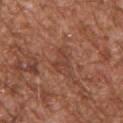Clinical impression: Imaged during a routine full-body skin examination; the lesion was not biopsied and no histopathology is available. Acquisition and patient details: A male subject, aged 43–47. The lesion's longest dimension is about 3 mm. Cropped from a whole-body photographic skin survey; the tile spans about 15 mm. An algorithmic analysis of the crop reported an outline eccentricity of about 0.75 (0 = round, 1 = elongated). And it measured border irregularity of about 6.5 on a 0–10 scale, a within-lesion color-variation index near 1.5/10, and radial color variation of about 0.5. The software also gave a nevus-likeness score of about 5/100 and a detector confidence of about 100 out of 100 that the crop contains a lesion. The lesion is located on the left upper arm.